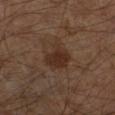* workup — no biopsy performed (imaged during a skin exam)
* site — the left lower leg
* subject — male, approximately 60 years of age
* lesion diameter — ~3.5 mm (longest diameter)
* imaging modality — ~15 mm tile from a whole-body skin photo